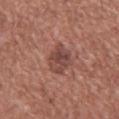Impression: Imaged during a routine full-body skin examination; the lesion was not biopsied and no histopathology is available. Clinical summary: A male patient, roughly 60 years of age. The lesion is on the back. The tile uses white-light illumination. A roughly 15 mm field-of-view crop from a total-body skin photograph.